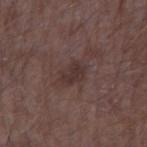workup = catalogued during a skin exam; not biopsied | patient = male, roughly 65 years of age | image source = ~15 mm tile from a whole-body skin photo | body site = the arm | automated lesion analysis = a mean CIELAB color near L≈32 a*≈15 b*≈17; a border-irregularity rating of about 3/10, internal color variation of about 1.5 on a 0–10 scale, and a peripheral color-asymmetry measure near 0.5 | diameter = about 3 mm | illumination = white-light illumination.